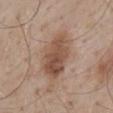size: ≈6 mm
tile lighting: white-light
patient: male, aged 53–57
image source: total-body-photography crop, ~15 mm field of view
location: the mid back